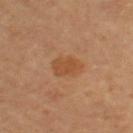workup = catalogued during a skin exam; not biopsied
acquisition = total-body-photography crop, ~15 mm field of view
patient = female, aged 63–67
site = the arm